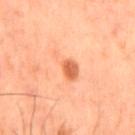Context: Automated tile analysis of the lesion measured a border-irregularity index near 4.5/10, internal color variation of about 4 on a 0–10 scale, and peripheral color asymmetry of about 1. It also reported a nevus-likeness score of about 80/100. A male patient approximately 60 years of age. This image is a 15 mm lesion crop taken from a total-body photograph. The tile uses cross-polarized illumination. The lesion is on the mid back.